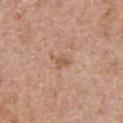Q: Was this lesion biopsied?
A: catalogued during a skin exam; not biopsied
Q: Automated lesion metrics?
A: an area of roughly 3.5 mm² and a shape eccentricity near 0.8; a mean CIELAB color near L≈57 a*≈20 b*≈32, a lesion–skin lightness drop of about 8, and a normalized border contrast of about 6; a border-irregularity index near 3.5/10 and a color-variation rating of about 1.5/10; a detector confidence of about 100 out of 100 that the crop contains a lesion
Q: What is the imaging modality?
A: 15 mm crop, total-body photography
Q: What is the anatomic site?
A: the chest
Q: Patient demographics?
A: male, roughly 70 years of age
Q: How was the tile lit?
A: white-light